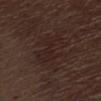Assessment:
The lesion was photographed on a routine skin check and not biopsied; there is no pathology result.